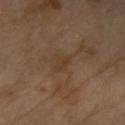– notes — total-body-photography surveillance lesion; no biopsy
– automated metrics — a border-irregularity rating of about 3/10 and peripheral color asymmetry of about 0.5; a nevus-likeness score of about 0/100
– illumination — cross-polarized illumination
– image — 15 mm crop, total-body photography
– diameter — ≈2.5 mm
– patient — female, aged 68–72
– site — the right forearm